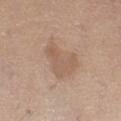The lesion was tiled from a total-body skin photograph and was not biopsied.
The recorded lesion diameter is about 5.5 mm.
The subject is a female aged around 60.
The lesion is on the left thigh.
An algorithmic analysis of the crop reported an average lesion color of about L≈58 a*≈17 b*≈28 (CIELAB). The software also gave a lesion-detection confidence of about 100/100.
A 15 mm close-up tile from a total-body photography series done for melanoma screening.
The tile uses white-light illumination.